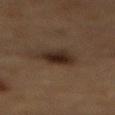{"biopsy_status": "not biopsied; imaged during a skin examination", "lesion_size": {"long_diameter_mm_approx": 3.5}, "image": {"source": "total-body photography crop", "field_of_view_mm": 15}, "automated_metrics": {"cielab_L": 22, "cielab_a": 13, "cielab_b": 20, "vs_skin_darker_L": 9.0, "vs_skin_contrast_norm": 11.0, "border_irregularity_0_10": 1.5, "peripheral_color_asymmetry": 1.0}, "site": "back", "patient": {"sex": "male", "age_approx": "85+"}, "lighting": "cross-polarized"}